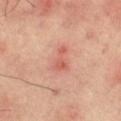Part of a total-body skin-imaging series; this lesion was reviewed on a skin check and was not flagged for biopsy. From the right thigh. This is a cross-polarized tile. About 3.5 mm across. Cropped from a total-body skin-imaging series; the visible field is about 15 mm.